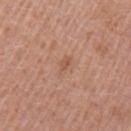biopsy status: no biopsy performed (imaged during a skin exam).